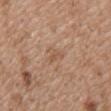Captured during whole-body skin photography for melanoma surveillance; the lesion was not biopsied. A male patient aged approximately 50. Imaged with white-light lighting. Measured at roughly 3 mm in maximum diameter. A 15 mm close-up tile from a total-body photography series done for melanoma screening. The lesion is located on the chest.